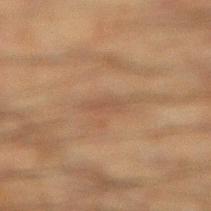No biopsy was performed on this lesion — it was imaged during a full skin examination and was not determined to be concerning.
A roughly 15 mm field-of-view crop from a total-body skin photograph.
A male subject in their mid-30s.
Located on the right lower leg.